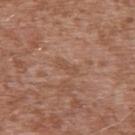subject = male, aged 43–47 | imaging modality = ~15 mm crop, total-body skin-cancer survey | TBP lesion metrics = border irregularity of about 4 on a 0–10 scale, internal color variation of about 0 on a 0–10 scale, and radial color variation of about 0 | anatomic site = the upper back | diameter = ~2.5 mm (longest diameter).